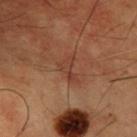Case summary:
* follow-up · total-body-photography surveillance lesion; no biopsy
* imaging modality · total-body-photography crop, ~15 mm field of view
* patient · male, aged around 50
* lesion size · about 3 mm
* anatomic site · the chest
* tile lighting · cross-polarized illumination